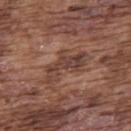<tbp_lesion>
  <biopsy_status>not biopsied; imaged during a skin examination</biopsy_status>
  <lighting>white-light</lighting>
  <image>
    <source>total-body photography crop</source>
    <field_of_view_mm>15</field_of_view_mm>
  </image>
  <patient>
    <sex>male</sex>
    <age_approx>75</age_approx>
  </patient>
  <site>upper back</site>
</tbp_lesion>